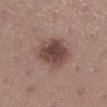Q: Was a biopsy performed?
A: imaged on a skin check; not biopsied